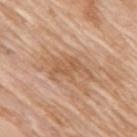follow-up=imaged on a skin check; not biopsied | tile lighting=white-light illumination | subject=female, aged around 75 | image=~15 mm tile from a whole-body skin photo | image-analysis metrics=radial color variation of about 1 | body site=the right upper arm.